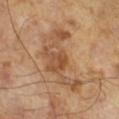The lesion was photographed on a routine skin check and not biopsied; there is no pathology result.
Imaged with cross-polarized lighting.
A 15 mm close-up tile from a total-body photography series done for melanoma screening.
Automated tile analysis of the lesion measured a lesion area of about 17 mm², an outline eccentricity of about 0.85 (0 = round, 1 = elongated), and a shape-asymmetry score of about 0.65 (0 = symmetric). And it measured an average lesion color of about L≈49 a*≈20 b*≈33 (CIELAB), about 9 CIELAB-L* units darker than the surrounding skin, and a normalized border contrast of about 7.
The recorded lesion diameter is about 7.5 mm.
A male patient about 65 years old.